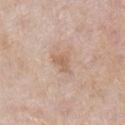automated metrics: an area of roughly 3 mm², an outline eccentricity of about 0.85 (0 = round, 1 = elongated), and a shape-asymmetry score of about 0.45 (0 = symmetric); an average lesion color of about L≈60 a*≈19 b*≈30 (CIELAB), about 8 CIELAB-L* units darker than the surrounding skin, and a normalized lesion–skin contrast near 6
size: ≈2.5 mm
location: the right lower leg
tile lighting: white-light
subject: female, roughly 60 years of age
acquisition: ~15 mm tile from a whole-body skin photo